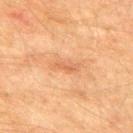Q: What is the imaging modality?
A: 15 mm crop, total-body photography
Q: How was the tile lit?
A: cross-polarized
Q: Automated lesion metrics?
A: border irregularity of about 4 on a 0–10 scale, a within-lesion color-variation index near 1.5/10, and radial color variation of about 0.5
Q: Lesion location?
A: the upper back
Q: What are the patient's age and sex?
A: male, aged around 75